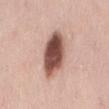Impression:
The lesion was tiled from a total-body skin photograph and was not biopsied.
Context:
This is a white-light tile. A 15 mm crop from a total-body photograph taken for skin-cancer surveillance. The total-body-photography lesion software estimated an automated nevus-likeness rating near 100 out of 100 and lesion-presence confidence of about 100/100. The lesion's longest dimension is about 6.5 mm. The subject is a male about 40 years old. The lesion is on the mid back.